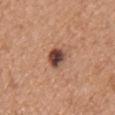This lesion was catalogued during total-body skin photography and was not selected for biopsy.
The lesion-visualizer software estimated a border-irregularity rating of about 2/10, a color-variation rating of about 5.5/10, and a peripheral color-asymmetry measure near 1.5. The analysis additionally found an automated nevus-likeness rating near 70 out of 100.
This image is a 15 mm lesion crop taken from a total-body photograph.
The lesion is on the chest.
A female patient, aged approximately 35.
Approximately 3 mm at its widest.